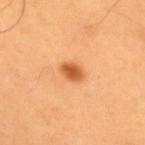Assessment: Captured during whole-body skin photography for melanoma surveillance; the lesion was not biopsied. Background: A region of skin cropped from a whole-body photographic capture, roughly 15 mm wide. The lesion is located on the upper back. A male patient about 55 years old. The tile uses cross-polarized illumination. About 2.5 mm across.